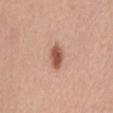This lesion was catalogued during total-body skin photography and was not selected for biopsy. The lesion is on the mid back. A 15 mm close-up extracted from a 3D total-body photography capture. A female subject, aged around 60. The lesion-visualizer software estimated a lesion area of about 5.5 mm², an eccentricity of roughly 0.8, and two-axis asymmetry of about 0.25. The analysis additionally found a lesion color around L≈55 a*≈23 b*≈31 in CIELAB, about 14 CIELAB-L* units darker than the surrounding skin, and a normalized lesion–skin contrast near 9.5. The software also gave an automated nevus-likeness rating near 100 out of 100 and a detector confidence of about 100 out of 100 that the crop contains a lesion.